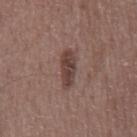location=the chest | image source=~15 mm tile from a whole-body skin photo | lighting=white-light | subject=male, in their 60s.